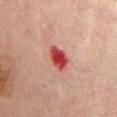Captured during whole-body skin photography for melanoma surveillance; the lesion was not biopsied. Cropped from a whole-body photographic skin survey; the tile spans about 15 mm. A female patient aged 58 to 62. The lesion is located on the front of the torso. Captured under cross-polarized illumination. The lesion's longest dimension is about 3.5 mm.